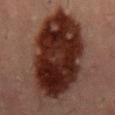  biopsy_status: not biopsied; imaged during a skin examination
  image:
    source: total-body photography crop
    field_of_view_mm: 15
  site: front of the torso
  patient:
    sex: male
    age_approx: 55